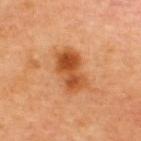follow-up: no biopsy performed (imaged during a skin exam); tile lighting: cross-polarized; subject: male, aged 43–47; site: the upper back; acquisition: total-body-photography crop, ~15 mm field of view.